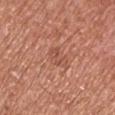The lesion was photographed on a routine skin check and not biopsied; there is no pathology result. The recorded lesion diameter is about 3 mm. The lesion is on the right upper arm. A male patient, aged around 65. Captured under white-light illumination. A 15 mm crop from a total-body photograph taken for skin-cancer surveillance.